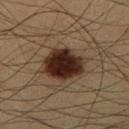biopsy status=no biopsy performed (imaged during a skin exam)
patient=male, roughly 35 years of age
image source=~15 mm crop, total-body skin-cancer survey
diameter=~5.5 mm (longest diameter)
TBP lesion metrics=an eccentricity of roughly 0.65 and a symmetry-axis asymmetry near 0.2; a lesion–skin lightness drop of about 16 and a normalized lesion–skin contrast near 16.5; a border-irregularity index near 2/10 and radial color variation of about 1.5
location=the right lower leg
illumination=cross-polarized illumination